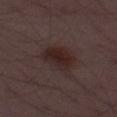Q: Is there a histopathology result?
A: catalogued during a skin exam; not biopsied
Q: Who is the patient?
A: male, aged 48–52
Q: What did automated image analysis measure?
A: a lesion color around L≈23 a*≈15 b*≈16 in CIELAB, about 7 CIELAB-L* units darker than the surrounding skin, and a lesion-to-skin contrast of about 9 (normalized; higher = more distinct); a nevus-likeness score of about 90/100 and lesion-presence confidence of about 100/100
Q: Lesion size?
A: about 4 mm
Q: How was this image acquired?
A: ~15 mm tile from a whole-body skin photo
Q: What lighting was used for the tile?
A: white-light